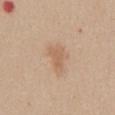Impression: Imaged during a routine full-body skin examination; the lesion was not biopsied and no histopathology is available. Context: The subject is a female aged around 40. A lesion tile, about 15 mm wide, cut from a 3D total-body photograph. From the abdomen.